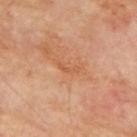image source = 15 mm crop, total-body photography
image-analysis metrics = an area of roughly 2 mm², an eccentricity of roughly 0.95, and two-axis asymmetry of about 0.55; a mean CIELAB color near L≈55 a*≈25 b*≈37, a lesion–skin lightness drop of about 6, and a normalized border contrast of about 5; a border-irregularity index near 6/10 and peripheral color asymmetry of about 0; a classifier nevus-likeness of about 0/100 and a lesion-detection confidence of about 95/100
lesion diameter = ~2.5 mm (longest diameter)
anatomic site = the mid back
lighting = cross-polarized illumination
patient = male, aged approximately 70